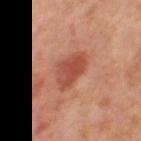Assessment:
The lesion was tiled from a total-body skin photograph and was not biopsied.
Background:
The total-body-photography lesion software estimated an outline eccentricity of about 0.8 (0 = round, 1 = elongated). It also reported border irregularity of about 3 on a 0–10 scale, internal color variation of about 4 on a 0–10 scale, and radial color variation of about 1.5. A female subject in their mid-50s. A 15 mm close-up tile from a total-body photography series done for melanoma screening. The lesion is on the right thigh. Longest diameter approximately 4.5 mm.